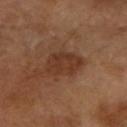Captured during whole-body skin photography for melanoma surveillance; the lesion was not biopsied. Located on the left forearm. About 4.5 mm across. This is a cross-polarized tile. This image is a 15 mm lesion crop taken from a total-body photograph. The patient is a female aged approximately 70.